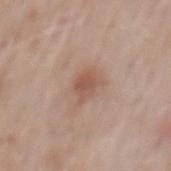<tbp_lesion>
<biopsy_status>not biopsied; imaged during a skin examination</biopsy_status>
<site>mid back</site>
<lighting>white-light</lighting>
<patient>
  <sex>male</sex>
  <age_approx>60</age_approx>
</patient>
<image>
  <source>total-body photography crop</source>
  <field_of_view_mm>15</field_of_view_mm>
</image>
<lesion_size>
  <long_diameter_mm_approx>2.5</long_diameter_mm_approx>
</lesion_size>
</tbp_lesion>